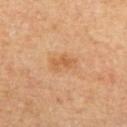* follow-up · total-body-photography surveillance lesion; no biopsy
* acquisition · 15 mm crop, total-body photography
* anatomic site · the left upper arm
* patient · male, in their mid-60s
* lighting · cross-polarized
* diameter · about 3.5 mm